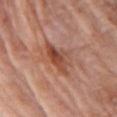Notes:
• location — the right upper arm
• patient — female, aged 83 or over
• acquisition — total-body-photography crop, ~15 mm field of view
• size — ≈5 mm
• automated metrics — a lesion area of about 7.5 mm², a shape eccentricity near 0.85, and a shape-asymmetry score of about 0.45 (0 = symmetric); a lesion–skin lightness drop of about 11 and a lesion-to-skin contrast of about 8.5 (normalized; higher = more distinct)